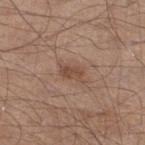Context:
A male patient approximately 55 years of age. On the left lower leg. Imaged with white-light lighting. The total-body-photography lesion software estimated a footprint of about 4 mm², a shape eccentricity near 0.8, and a symmetry-axis asymmetry near 0.3. And it measured a border-irregularity rating of about 3/10, a within-lesion color-variation index near 2/10, and peripheral color asymmetry of about 0.5. The analysis additionally found an automated nevus-likeness rating near 30 out of 100. Cropped from a whole-body photographic skin survey; the tile spans about 15 mm.